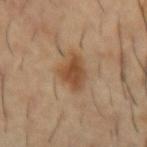biopsy status: imaged on a skin check; not biopsied | lesion size: ~3.5 mm (longest diameter) | imaging modality: 15 mm crop, total-body photography | patient: male, aged around 55 | location: the right forearm.